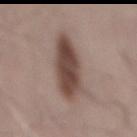Captured during whole-body skin photography for melanoma surveillance; the lesion was not biopsied. A close-up tile cropped from a whole-body skin photograph, about 15 mm across. Located on the mid back. The recorded lesion diameter is about 6.5 mm. The patient is a male aged around 70. Captured under white-light illumination.